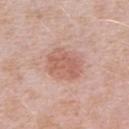A 15 mm close-up extracted from a 3D total-body photography capture. Measured at roughly 4.5 mm in maximum diameter. Captured under white-light illumination. A female subject, in their mid- to late 40s. The lesion is located on the right upper arm.